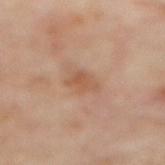Imaged during a routine full-body skin examination; the lesion was not biopsied and no histopathology is available.
Longest diameter approximately 3 mm.
The patient is a female aged 58 to 62.
A lesion tile, about 15 mm wide, cut from a 3D total-body photograph.
Imaged with cross-polarized lighting.
From the mid back.
The lesion-visualizer software estimated an average lesion color of about L≈54 a*≈21 b*≈32 (CIELAB), about 8 CIELAB-L* units darker than the surrounding skin, and a normalized lesion–skin contrast near 6. The analysis additionally found a nevus-likeness score of about 0/100 and a detector confidence of about 100 out of 100 that the crop contains a lesion.